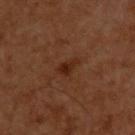Assessment: Part of a total-body skin-imaging series; this lesion was reviewed on a skin check and was not flagged for biopsy. Acquisition and patient details: This is a cross-polarized tile. A male subject, aged around 60. Located on the upper back. The lesion-visualizer software estimated a border-irregularity index near 4/10, a within-lesion color-variation index near 1/10, and a peripheral color-asymmetry measure near 0.5. This image is a 15 mm lesion crop taken from a total-body photograph.